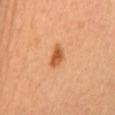Part of a total-body skin-imaging series; this lesion was reviewed on a skin check and was not flagged for biopsy. The recorded lesion diameter is about 3 mm. A region of skin cropped from a whole-body photographic capture, roughly 15 mm wide. The lesion is located on the mid back. An algorithmic analysis of the crop reported an average lesion color of about L≈57 a*≈29 b*≈42 (CIELAB), about 13 CIELAB-L* units darker than the surrounding skin, and a lesion-to-skin contrast of about 9 (normalized; higher = more distinct). The software also gave a classifier nevus-likeness of about 95/100 and a detector confidence of about 100 out of 100 that the crop contains a lesion. A female subject.